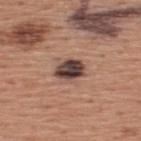The lesion was photographed on a routine skin check and not biopsied; there is no pathology result.
Captured under white-light illumination.
A 15 mm close-up extracted from a 3D total-body photography capture.
A male subject, approximately 45 years of age.
Located on the upper back.